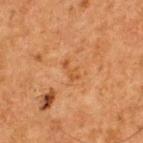Clinical summary:
The lesion is on the upper back. The subject is a male aged approximately 65. A 15 mm close-up tile from a total-body photography series done for melanoma screening.